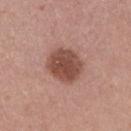workup: catalogued during a skin exam; not biopsied
image: total-body-photography crop, ~15 mm field of view
patient: female, approximately 50 years of age
location: the right upper arm